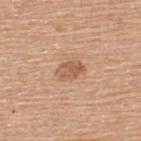A region of skin cropped from a whole-body photographic capture, roughly 15 mm wide.
A female subject aged 58–62.
The lesion is located on the upper back.